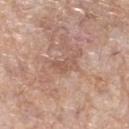No biopsy was performed on this lesion — it was imaged during a full skin examination and was not determined to be concerning.
A lesion tile, about 15 mm wide, cut from a 3D total-body photograph.
Located on the left lower leg.
A female subject approximately 70 years of age.